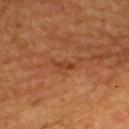Recorded during total-body skin imaging; not selected for excision or biopsy.
The recorded lesion diameter is about 3 mm.
From the upper back.
A roughly 15 mm field-of-view crop from a total-body skin photograph.
A male subject, aged 63–67.
Captured under cross-polarized illumination.